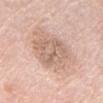Findings:
– biopsy status · imaged on a skin check; not biopsied
– site · the abdomen
– lighting · white-light
– TBP lesion metrics · an area of roughly 15 mm², an outline eccentricity of about 0.5 (0 = round, 1 = elongated), and two-axis asymmetry of about 0.2; a lesion color around L≈64 a*≈18 b*≈28 in CIELAB, roughly 8 lightness units darker than nearby skin, and a normalized border contrast of about 5.5; lesion-presence confidence of about 100/100
– image source · ~15 mm crop, total-body skin-cancer survey
– patient · male, in their 80s